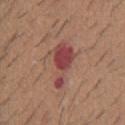No biopsy was performed on this lesion — it was imaged during a full skin examination and was not determined to be concerning.
A male subject, roughly 60 years of age.
The tile uses white-light illumination.
On the mid back.
A 15 mm close-up extracted from a 3D total-body photography capture.
The lesion-visualizer software estimated an average lesion color of about L≈44 a*≈29 b*≈23 (CIELAB), roughly 12 lightness units darker than nearby skin, and a normalized lesion–skin contrast near 9.5. And it measured a classifier nevus-likeness of about 0/100 and a detector confidence of about 100 out of 100 that the crop contains a lesion.